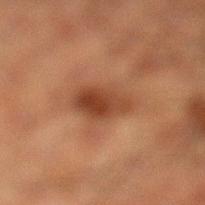workup: no biopsy performed (imaged during a skin exam) | tile lighting: cross-polarized | subject: male, in their 50s | body site: the left lower leg | diameter: about 5 mm | imaging modality: total-body-photography crop, ~15 mm field of view.